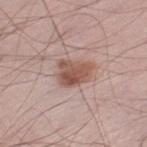patient = male, aged 53 to 57 | lesion size = ~4 mm (longest diameter) | anatomic site = the left thigh | tile lighting = white-light illumination | imaging modality = 15 mm crop, total-body photography | automated metrics = a color-variation rating of about 4.5/10 and peripheral color asymmetry of about 1.5; a nevus-likeness score of about 95/100 and a detector confidence of about 100 out of 100 that the crop contains a lesion.